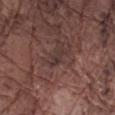Findings:
- follow-up — imaged on a skin check; not biopsied
- subject — male, approximately 75 years of age
- diameter — ~2.5 mm (longest diameter)
- location — the arm
- acquisition — ~15 mm crop, total-body skin-cancer survey
- lighting — white-light